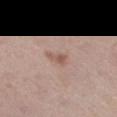Part of a total-body skin-imaging series; this lesion was reviewed on a skin check and was not flagged for biopsy.
The tile uses white-light illumination.
The patient is a male aged 73 to 77.
Approximately 3 mm at its widest.
A lesion tile, about 15 mm wide, cut from a 3D total-body photograph.
The lesion is located on the left thigh.